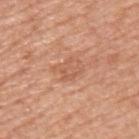The lesion was photographed on a routine skin check and not biopsied; there is no pathology result.
The lesion-visualizer software estimated a lesion area of about 7.5 mm², a shape eccentricity near 0.75, and a shape-asymmetry score of about 0.25 (0 = symmetric). The software also gave roughly 7 lightness units darker than nearby skin. It also reported a nevus-likeness score of about 0/100 and a detector confidence of about 100 out of 100 that the crop contains a lesion.
A 15 mm close-up extracted from a 3D total-body photography capture.
On the upper back.
The subject is a male aged around 50.
The lesion's longest dimension is about 3.5 mm.